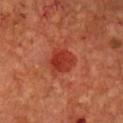  biopsy_status: not biopsied; imaged during a skin examination
  site: chest
  patient:
    sex: male
    age_approx: 50
  lighting: cross-polarized
  image:
    source: total-body photography crop
    field_of_view_mm: 15